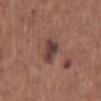{
  "lighting": "white-light",
  "lesion_size": {
    "long_diameter_mm_approx": 3.5
  },
  "automated_metrics": {
    "cielab_L": 40,
    "cielab_a": 21,
    "cielab_b": 21,
    "vs_skin_contrast_norm": 9.5,
    "nevus_likeness_0_100": 25,
    "lesion_detection_confidence_0_100": 100
  },
  "patient": {
    "sex": "male",
    "age_approx": 75
  },
  "site": "chest",
  "image": {
    "source": "total-body photography crop",
    "field_of_view_mm": 15
  }
}